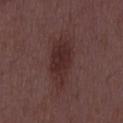Assessment: Recorded during total-body skin imaging; not selected for excision or biopsy. Clinical summary: A 15 mm crop from a total-body photograph taken for skin-cancer surveillance. An algorithmic analysis of the crop reported internal color variation of about 3 on a 0–10 scale and a peripheral color-asymmetry measure near 1. Captured under white-light illumination. The subject is a male aged 48–52.